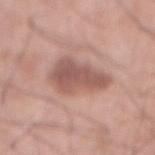Cropped from a total-body skin-imaging series; the visible field is about 15 mm. A male subject aged approximately 70. From the abdomen.